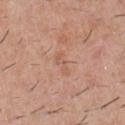No biopsy was performed on this lesion — it was imaged during a full skin examination and was not determined to be concerning. The recorded lesion diameter is about 3 mm. The total-body-photography lesion software estimated an automated nevus-likeness rating near 0 out of 100. Captured under white-light illumination. The patient is a male aged approximately 30. A 15 mm crop from a total-body photograph taken for skin-cancer surveillance. The lesion is located on the chest.